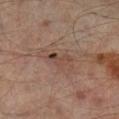Q: Was this lesion biopsied?
A: imaged on a skin check; not biopsied
Q: Who is the patient?
A: male, in their mid- to late 60s
Q: Where on the body is the lesion?
A: the right lower leg
Q: What is the imaging modality?
A: ~15 mm crop, total-body skin-cancer survey